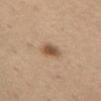The lesion was photographed on a routine skin check and not biopsied; there is no pathology result.
A male subject, in their 70s.
A 15 mm close-up extracted from a 3D total-body photography capture.
Located on the abdomen.
An algorithmic analysis of the crop reported a lesion area of about 5.5 mm², a shape eccentricity near 0.85, and a symmetry-axis asymmetry near 0.25. It also reported an automated nevus-likeness rating near 95 out of 100 and lesion-presence confidence of about 100/100.